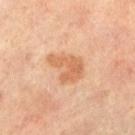The lesion was tiled from a total-body skin photograph and was not biopsied.